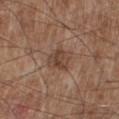Case summary:
- workup · total-body-photography surveillance lesion; no biopsy
- patient · male, aged approximately 55
- tile lighting · white-light illumination
- body site · the right lower leg
- lesion diameter · ~3 mm (longest diameter)
- acquisition · ~15 mm tile from a whole-body skin photo
- TBP lesion metrics · a mean CIELAB color near L≈43 a*≈18 b*≈26, about 9 CIELAB-L* units darker than the surrounding skin, and a normalized border contrast of about 7; an automated nevus-likeness rating near 0 out of 100 and lesion-presence confidence of about 100/100